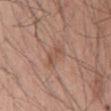Assessment: This lesion was catalogued during total-body skin photography and was not selected for biopsy. Context: From the chest. Imaged with white-light lighting. Cropped from a total-body skin-imaging series; the visible field is about 15 mm. Measured at roughly 3 mm in maximum diameter. A male subject in their mid-50s. Automated image analysis of the tile measured an eccentricity of roughly 0.75. It also reported an automated nevus-likeness rating near 0 out of 100 and a lesion-detection confidence of about 100/100.